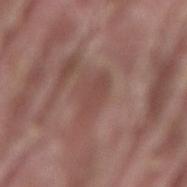Imaged during a routine full-body skin examination; the lesion was not biopsied and no histopathology is available. About 3.5 mm across. Automated tile analysis of the lesion measured an average lesion color of about L≈46 a*≈21 b*≈23 (CIELAB) and a lesion-to-skin contrast of about 5 (normalized; higher = more distinct). And it measured a within-lesion color-variation index near 2/10. A 15 mm close-up tile from a total-body photography series done for melanoma screening. A male patient roughly 40 years of age. Imaged with white-light lighting. On the lower back.